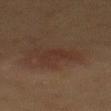A 15 mm close-up extracted from a 3D total-body photography capture. The lesion is located on the back. A female patient roughly 50 years of age. About 3 mm across.